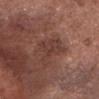Findings:
• patient: male, aged approximately 75
• image-analysis metrics: an area of roughly 9 mm² and an outline eccentricity of about 0.8 (0 = round, 1 = elongated); a border-irregularity rating of about 6.5/10, internal color variation of about 2 on a 0–10 scale, and peripheral color asymmetry of about 1
• lesion diameter: about 5 mm
• lighting: white-light
• acquisition: 15 mm crop, total-body photography
• site: the head or neck